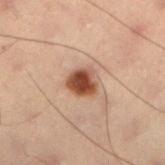Q: Was a biopsy performed?
A: total-body-photography surveillance lesion; no biopsy
Q: What is the imaging modality?
A: ~15 mm tile from a whole-body skin photo
Q: Lesion location?
A: the left lower leg
Q: What did automated image analysis measure?
A: a lesion area of about 7 mm², a shape eccentricity near 0.45, and two-axis asymmetry of about 0.2
Q: What are the patient's age and sex?
A: male, in their mid-50s
Q: Illumination type?
A: cross-polarized
Q: What is the lesion's diameter?
A: about 3 mm